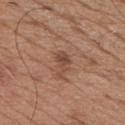Q: Was a biopsy performed?
A: total-body-photography surveillance lesion; no biopsy
Q: Who is the patient?
A: male, roughly 65 years of age
Q: How was the tile lit?
A: white-light illumination
Q: What is the anatomic site?
A: the chest
Q: How was this image acquired?
A: ~15 mm crop, total-body skin-cancer survey
Q: What is the lesion's diameter?
A: ~3 mm (longest diameter)